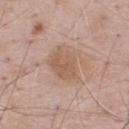workup: no biopsy performed (imaged during a skin exam) | automated lesion analysis: an area of roughly 12 mm² and a shape eccentricity near 0.5; a lesion–skin lightness drop of about 8 and a lesion-to-skin contrast of about 6 (normalized; higher = more distinct); a detector confidence of about 100 out of 100 that the crop contains a lesion | image: ~15 mm crop, total-body skin-cancer survey | location: the upper back | subject: male, aged 68 to 72 | tile lighting: white-light illumination.